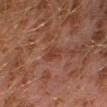Recorded during total-body skin imaging; not selected for excision or biopsy. A 15 mm crop from a total-body photograph taken for skin-cancer surveillance. The subject is a male approximately 30 years of age. On the left leg.